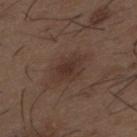<lesion>
  <biopsy_status>not biopsied; imaged during a skin examination</biopsy_status>
  <lighting>white-light</lighting>
  <patient>
    <sex>male</sex>
    <age_approx>50</age_approx>
  </patient>
  <image>
    <source>total-body photography crop</source>
    <field_of_view_mm>15</field_of_view_mm>
  </image>
  <lesion_size>
    <long_diameter_mm_approx>4.5</long_diameter_mm_approx>
  </lesion_size>
  <automated_metrics>
    <area_mm2_approx>9.0</area_mm2_approx>
    <shape_asymmetry>0.2</shape_asymmetry>
    <nevus_likeness_0_100>20</nevus_likeness_0_100>
  </automated_metrics>
  <site>mid back</site>
</lesion>